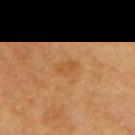Impression: Imaged during a routine full-body skin examination; the lesion was not biopsied and no histopathology is available. Acquisition and patient details: The recorded lesion diameter is about 2.5 mm. The subject is a female aged 58–62. A 15 mm close-up tile from a total-body photography series done for melanoma screening. Located on the left arm. Captured under cross-polarized illumination.